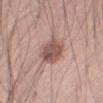On the right thigh. Cropped from a total-body skin-imaging series; the visible field is about 15 mm. The patient is a male in their mid-50s.